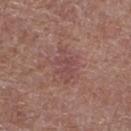No biopsy was performed on this lesion — it was imaged during a full skin examination and was not determined to be concerning. Imaged with white-light lighting. On the right lower leg. The subject is a male aged 68–72. A lesion tile, about 15 mm wide, cut from a 3D total-body photograph.